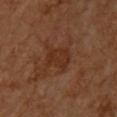{"biopsy_status": "not biopsied; imaged during a skin examination", "image": {"source": "total-body photography crop", "field_of_view_mm": 15}, "lighting": "cross-polarized", "patient": {"sex": "male", "age_approx": 60}, "site": "upper back", "lesion_size": {"long_diameter_mm_approx": 3.5}}